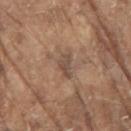Image and clinical context:
A 15 mm close-up extracted from a 3D total-body photography capture. The lesion's longest dimension is about 3 mm. From the arm. Imaged with white-light lighting. A male subject, aged 78 to 82.